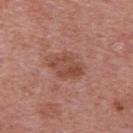Q: Was this lesion biopsied?
A: catalogued during a skin exam; not biopsied
Q: What is the imaging modality?
A: total-body-photography crop, ~15 mm field of view
Q: What is the anatomic site?
A: the upper back
Q: What lighting was used for the tile?
A: white-light illumination
Q: What are the patient's age and sex?
A: male, aged approximately 60
Q: What is the lesion's diameter?
A: ~3.5 mm (longest diameter)